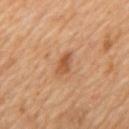A close-up tile cropped from a whole-body skin photograph, about 15 mm across. A male patient in their mid- to late 60s. On the upper back.